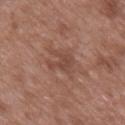The lesion was tiled from a total-body skin photograph and was not biopsied. The subject is a male aged around 50. A 15 mm close-up extracted from a 3D total-body photography capture. Longest diameter approximately 3 mm. This is a white-light tile. From the mid back.